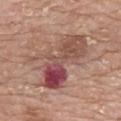{
  "biopsy_status": "not biopsied; imaged during a skin examination",
  "lesion_size": {
    "long_diameter_mm_approx": 8.5
  },
  "site": "chest",
  "automated_metrics": {
    "area_mm2_approx": 26.0,
    "shape_asymmetry": 0.5,
    "cielab_L": 50,
    "cielab_a": 23,
    "cielab_b": 24,
    "vs_skin_darker_L": 12.0,
    "vs_skin_contrast_norm": 8.5,
    "nevus_likeness_0_100": 0,
    "lesion_detection_confidence_0_100": 100
  },
  "image": {
    "source": "total-body photography crop",
    "field_of_view_mm": 15
  },
  "patient": {
    "sex": "female",
    "age_approx": 65
  }
}